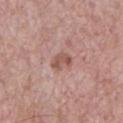Q: Was a biopsy performed?
A: imaged on a skin check; not biopsied
Q: What are the patient's age and sex?
A: male, roughly 55 years of age
Q: Illumination type?
A: white-light illumination
Q: What is the imaging modality?
A: total-body-photography crop, ~15 mm field of view
Q: What is the lesion's diameter?
A: ≈2.5 mm
Q: What did automated image analysis measure?
A: an area of roughly 4 mm² and two-axis asymmetry of about 0.3; an average lesion color of about L≈53 a*≈21 b*≈25 (CIELAB), roughly 10 lightness units darker than nearby skin, and a normalized border contrast of about 7